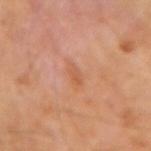| field | value |
|---|---|
| notes | imaged on a skin check; not biopsied |
| size | ≈2 mm |
| site | the left forearm |
| acquisition | ~15 mm tile from a whole-body skin photo |
| illumination | cross-polarized |
| automated metrics | an area of roughly 2.5 mm², an eccentricity of roughly 0.85, and a symmetry-axis asymmetry near 0.25; a border-irregularity rating of about 2/10, a within-lesion color-variation index near 1.5/10, and a peripheral color-asymmetry measure near 0.5; an automated nevus-likeness rating near 0 out of 100 and a lesion-detection confidence of about 100/100 |
| subject | female, aged around 60 |